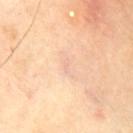Q: Was a biopsy performed?
A: no biopsy performed (imaged during a skin exam)
Q: What did automated image analysis measure?
A: an area of roughly 0.5 mm²; a border-irregularity rating of about 4/10 and a within-lesion color-variation index near 0/10
Q: How large is the lesion?
A: ≈1.5 mm
Q: Lesion location?
A: the front of the torso
Q: What kind of image is this?
A: ~15 mm tile from a whole-body skin photo
Q: How was the tile lit?
A: cross-polarized illumination
Q: Patient demographics?
A: male, in their 70s